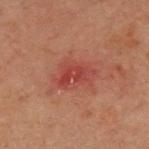Case summary:
* notes — catalogued during a skin exam; not biopsied
* acquisition — total-body-photography crop, ~15 mm field of view
* TBP lesion metrics — a border-irregularity index near 3/10, a within-lesion color-variation index near 4.5/10, and peripheral color asymmetry of about 1.5; a nevus-likeness score of about 0/100 and a lesion-detection confidence of about 100/100
* location — the chest
* tile lighting — cross-polarized illumination
* patient — male, aged 58 to 62